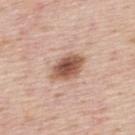biopsy status = catalogued during a skin exam; not biopsied
subject = male, in their mid-40s
body site = the upper back
acquisition = total-body-photography crop, ~15 mm field of view
lighting = white-light illumination